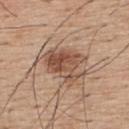Clinical impression: Part of a total-body skin-imaging series; this lesion was reviewed on a skin check and was not flagged for biopsy.